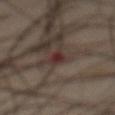The lesion is on the chest. A male patient, aged approximately 50. Cropped from a whole-body photographic skin survey; the tile spans about 15 mm.